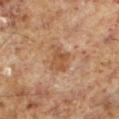The subject is a male aged 58 to 62.
The lesion's longest dimension is about 4 mm.
The tile uses cross-polarized illumination.
The total-body-photography lesion software estimated a lesion area of about 7 mm², a shape eccentricity near 0.75, and a shape-asymmetry score of about 0.35 (0 = symmetric). And it measured a mean CIELAB color near L≈49 a*≈20 b*≈33 and about 8 CIELAB-L* units darker than the surrounding skin. The analysis additionally found a border-irregularity index near 4.5/10. The software also gave a classifier nevus-likeness of about 0/100 and a detector confidence of about 100 out of 100 that the crop contains a lesion.
Located on the left lower leg.
Cropped from a total-body skin-imaging series; the visible field is about 15 mm.